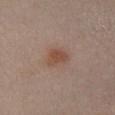Automated image analysis of the tile measured an outline eccentricity of about 0.45 (0 = round, 1 = elongated) and a symmetry-axis asymmetry near 0.3. The analysis additionally found a lesion color around L≈49 a*≈19 b*≈27 in CIELAB, about 8 CIELAB-L* units darker than the surrounding skin, and a lesion-to-skin contrast of about 7 (normalized; higher = more distinct). The software also gave a border-irregularity index near 2.5/10, a color-variation rating of about 3/10, and a peripheral color-asymmetry measure near 1. And it measured a nevus-likeness score of about 95/100. Measured at roughly 3 mm in maximum diameter. This image is a 15 mm lesion crop taken from a total-body photograph. Located on the chest. This is a white-light tile. A female subject in their 30s.